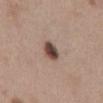A female subject aged around 40. Longest diameter approximately 3 mm. The tile uses white-light illumination. A close-up tile cropped from a whole-body skin photograph, about 15 mm across. Located on the abdomen.